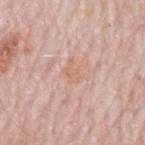notes=no biopsy performed (imaged during a skin exam) | location=the mid back | subject=male, aged 78–82 | diameter=about 2.5 mm | illumination=white-light | image=~15 mm tile from a whole-body skin photo | TBP lesion metrics=an average lesion color of about L≈65 a*≈20 b*≈31 (CIELAB) and about 6 CIELAB-L* units darker than the surrounding skin; a classifier nevus-likeness of about 0/100 and a detector confidence of about 100 out of 100 that the crop contains a lesion.